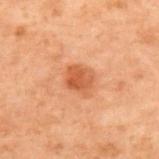Findings:
- biopsy status — catalogued during a skin exam; not biopsied
- automated metrics — a lesion area of about 7.5 mm², an eccentricity of roughly 0.5, and a shape-asymmetry score of about 0.2 (0 = symmetric); a mean CIELAB color near L≈44 a*≈23 b*≈32 and roughly 8 lightness units darker than nearby skin
- subject — male, about 70 years old
- acquisition — total-body-photography crop, ~15 mm field of view
- body site — the upper back
- size — ~3 mm (longest diameter)